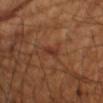Context:
On the left upper arm. A male subject, in their mid-60s. A region of skin cropped from a whole-body photographic capture, roughly 15 mm wide.